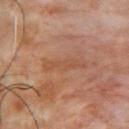biopsy status = total-body-photography surveillance lesion; no biopsy | subject = male, aged around 55 | anatomic site = the chest | size = ≈5 mm | image source = total-body-photography crop, ~15 mm field of view | image-analysis metrics = a border-irregularity index near 5/10, a within-lesion color-variation index near 1/10, and radial color variation of about 0; a nevus-likeness score of about 0/100 and lesion-presence confidence of about 90/100 | illumination = cross-polarized.